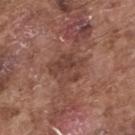Findings:
- follow-up: total-body-photography surveillance lesion; no biopsy
- tile lighting: white-light illumination
- location: the upper back
- image-analysis metrics: an area of roughly 10 mm²; a lesion color around L≈42 a*≈21 b*≈25 in CIELAB, roughly 8 lightness units darker than nearby skin, and a normalized border contrast of about 6.5; a border-irregularity index near 3.5/10, a color-variation rating of about 3.5/10, and peripheral color asymmetry of about 1.5; lesion-presence confidence of about 100/100
- subject: male, roughly 75 years of age
- lesion size: about 4.5 mm
- acquisition: ~15 mm tile from a whole-body skin photo